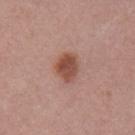Clinical impression:
This lesion was catalogued during total-body skin photography and was not selected for biopsy.
Acquisition and patient details:
The lesion is on the left upper arm. The patient is a female roughly 50 years of age. Cropped from a whole-body photographic skin survey; the tile spans about 15 mm.